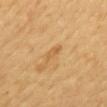Assessment: The lesion was tiled from a total-body skin photograph and was not biopsied. Background: A lesion tile, about 15 mm wide, cut from a 3D total-body photograph. Located on the mid back. A female patient aged 53–57. Approximately 2.5 mm at its widest.